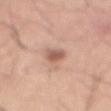Findings:
• notes — imaged on a skin check; not biopsied
• automated lesion analysis — a lesion area of about 4.5 mm², an outline eccentricity of about 0.8 (0 = round, 1 = elongated), and a shape-asymmetry score of about 0.3 (0 = symmetric); a mean CIELAB color near L≈58 a*≈21 b*≈28 and a normalized lesion–skin contrast near 7.5; an automated nevus-likeness rating near 85 out of 100 and a detector confidence of about 100 out of 100 that the crop contains a lesion
• lesion size — ~3 mm (longest diameter)
• tile lighting — white-light illumination
• location — the abdomen
• image source — 15 mm crop, total-body photography
• subject — male, aged 48 to 52